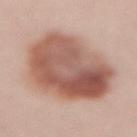pathology = a dysplastic (Clark) nevus